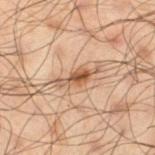Clinical impression: Recorded during total-body skin imaging; not selected for excision or biopsy. Background: About 4.5 mm across. From the left thigh. The lesion-visualizer software estimated a normalized lesion–skin contrast near 8.5. The software also gave a border-irregularity index near 4.5/10, internal color variation of about 3.5 on a 0–10 scale, and radial color variation of about 1.5. And it measured lesion-presence confidence of about 100/100. This image is a 15 mm lesion crop taken from a total-body photograph. This is a cross-polarized tile. A male patient roughly 50 years of age.